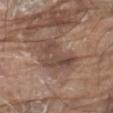The total-body-photography lesion software estimated an average lesion color of about L≈46 a*≈16 b*≈23 (CIELAB), roughly 9 lightness units darker than nearby skin, and a normalized lesion–skin contrast near 7. The analysis additionally found a border-irregularity index near 6.5/10, internal color variation of about 4.5 on a 0–10 scale, and a peripheral color-asymmetry measure near 2.
From the abdomen.
The patient is a male aged around 80.
Cropped from a total-body skin-imaging series; the visible field is about 15 mm.